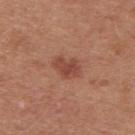Recorded during total-body skin imaging; not selected for excision or biopsy. An algorithmic analysis of the crop reported a footprint of about 6 mm², an outline eccentricity of about 0.75 (0 = round, 1 = elongated), and two-axis asymmetry of about 0.25. The software also gave a border-irregularity rating of about 2.5/10, internal color variation of about 2.5 on a 0–10 scale, and radial color variation of about 1. A male patient, aged 28–32. Measured at roughly 3.5 mm in maximum diameter. A close-up tile cropped from a whole-body skin photograph, about 15 mm across. Located on the upper back. Captured under white-light illumination.